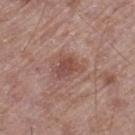Part of a total-body skin-imaging series; this lesion was reviewed on a skin check and was not flagged for biopsy. A male patient aged around 70. The lesion is on the left thigh. Automated image analysis of the tile measured a footprint of about 5 mm², a shape eccentricity near 0.7, and a shape-asymmetry score of about 0.35 (0 = symmetric). The software also gave about 9 CIELAB-L* units darker than the surrounding skin and a normalized lesion–skin contrast near 7. And it measured an automated nevus-likeness rating near 5 out of 100. Imaged with white-light lighting. The lesion's longest dimension is about 3 mm. A lesion tile, about 15 mm wide, cut from a 3D total-body photograph.